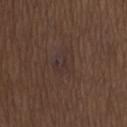{
  "patient": {
    "sex": "male",
    "age_approx": 70
  },
  "site": "mid back",
  "image": {
    "source": "total-body photography crop",
    "field_of_view_mm": 15
  },
  "lesion_size": {
    "long_diameter_mm_approx": 3.5
  }
}